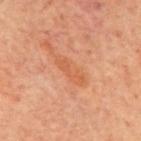Impression:
Part of a total-body skin-imaging series; this lesion was reviewed on a skin check and was not flagged for biopsy.
Background:
A 15 mm close-up extracted from a 3D total-body photography capture. The patient is a male aged 68–72. Located on the mid back. Captured under cross-polarized illumination. Approximately 4.5 mm at its widest. An algorithmic analysis of the crop reported an area of roughly 5.5 mm², an outline eccentricity of about 0.95 (0 = round, 1 = elongated), and two-axis asymmetry of about 0.3. It also reported a mean CIELAB color near L≈57 a*≈27 b*≈38 and roughly 6 lightness units darker than nearby skin. The software also gave a border-irregularity index near 4/10, a color-variation rating of about 1.5/10, and radial color variation of about 0.5. It also reported a classifier nevus-likeness of about 0/100 and a detector confidence of about 100 out of 100 that the crop contains a lesion.